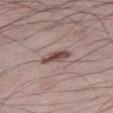Clinical impression:
Captured during whole-body skin photography for melanoma surveillance; the lesion was not biopsied.
Image and clinical context:
Cropped from a whole-body photographic skin survey; the tile spans about 15 mm. Measured at roughly 3.5 mm in maximum diameter. This is a white-light tile. Located on the right thigh. The patient is a male in their 70s.